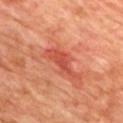This lesion was catalogued during total-body skin photography and was not selected for biopsy. The lesion-visualizer software estimated an area of roughly 7 mm² and a shape-asymmetry score of about 0.4 (0 = symmetric). And it measured a lesion color around L≈51 a*≈36 b*≈35 in CIELAB and a lesion–skin lightness drop of about 9. A male patient, about 70 years old. The lesion's longest dimension is about 5 mm. Captured under cross-polarized illumination. A 15 mm crop from a total-body photograph taken for skin-cancer surveillance. The lesion is located on the chest.